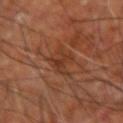* follow-up · catalogued during a skin exam; not biopsied
* subject · aged 63–67
* body site · the right upper arm
* image · ~15 mm crop, total-body skin-cancer survey